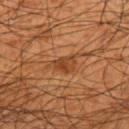Recorded during total-body skin imaging; not selected for excision or biopsy. Cropped from a total-body skin-imaging series; the visible field is about 15 mm. This is a cross-polarized tile. A male subject, aged 43 to 47. Located on the left upper arm. Longest diameter approximately 3.5 mm.